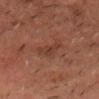<case>
<automated_metrics>
  <cielab_L>29</cielab_L>
  <cielab_a>18</cielab_a>
  <cielab_b>23</cielab_b>
  <vs_skin_darker_L>5.0</vs_skin_darker_L>
  <vs_skin_contrast_norm>5.5</vs_skin_contrast_norm>
  <nevus_likeness_0_100>0</nevus_likeness_0_100>
  <lesion_detection_confidence_0_100>100</lesion_detection_confidence_0_100>
</automated_metrics>
<lesion_size>
  <long_diameter_mm_approx>3.0</long_diameter_mm_approx>
</lesion_size>
<site>head or neck</site>
<image>
  <source>total-body photography crop</source>
  <field_of_view_mm>15</field_of_view_mm>
</image>
<lighting>cross-polarized</lighting>
<patient>
  <sex>male</sex>
  <age_approx>65</age_approx>
</patient>
</case>